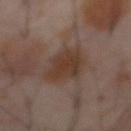Captured during whole-body skin photography for melanoma surveillance; the lesion was not biopsied.
A 15 mm close-up tile from a total-body photography series done for melanoma screening.
The lesion's longest dimension is about 4.5 mm.
The subject is a male aged approximately 60.
From the abdomen.
Imaged with cross-polarized lighting.